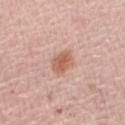biopsy status=no biopsy performed (imaged during a skin exam) | patient=female, aged 63 to 67 | anatomic site=the right forearm | image=total-body-photography crop, ~15 mm field of view | diameter=about 3 mm | illumination=white-light illumination | TBP lesion metrics=a mean CIELAB color near L≈60 a*≈22 b*≈30, about 11 CIELAB-L* units darker than the surrounding skin, and a normalized border contrast of about 8.